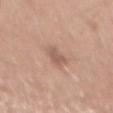Notes:
• biopsy status: imaged on a skin check; not biopsied